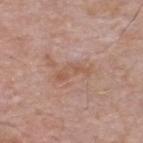Context: Located on the upper back. A male patient, aged around 65. Captured under white-light illumination. A close-up tile cropped from a whole-body skin photograph, about 15 mm across. About 4 mm across.